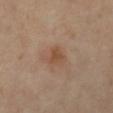This lesion was catalogued during total-body skin photography and was not selected for biopsy.
A female patient, roughly 60 years of age.
From the right leg.
A close-up tile cropped from a whole-body skin photograph, about 15 mm across.
This is a cross-polarized tile.
Measured at roughly 2.5 mm in maximum diameter.
Automated tile analysis of the lesion measured a lesion area of about 4 mm², a shape eccentricity near 0.65, and a shape-asymmetry score of about 0.35 (0 = symmetric). The software also gave an average lesion color of about L≈41 a*≈16 b*≈27 (CIELAB) and a lesion-to-skin contrast of about 6.5 (normalized; higher = more distinct). And it measured internal color variation of about 3 on a 0–10 scale. The software also gave a nevus-likeness score of about 5/100.